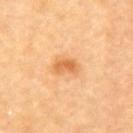biopsy status: catalogued during a skin exam; not biopsied | image-analysis metrics: a lesion area of about 5.5 mm² and a shape eccentricity near 0.8; border irregularity of about 3 on a 0–10 scale and a peripheral color-asymmetry measure near 1; a classifier nevus-likeness of about 75/100 | size: ~3 mm (longest diameter) | tile lighting: cross-polarized illumination | patient: male, aged approximately 85 or older | image: ~15 mm crop, total-body skin-cancer survey | body site: the arm.